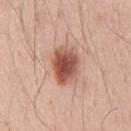  biopsy_status: not biopsied; imaged during a skin examination
  patient:
    sex: male
    age_approx: 45
  automated_metrics:
    cielab_L: 54
    cielab_a: 25
    cielab_b: 30
    vs_skin_darker_L: 16.0
    peripheral_color_asymmetry: 1.5
  lesion_size:
    long_diameter_mm_approx: 4.5
  lighting: white-light
  site: chest
  image:
    source: total-body photography crop
    field_of_view_mm: 15